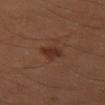follow-up: imaged on a skin check; not biopsied
subject: male, aged approximately 55
acquisition: 15 mm crop, total-body photography
lesion diameter: ≈3 mm
automated lesion analysis: a footprint of about 5 mm², a shape eccentricity near 0.5, and a shape-asymmetry score of about 0.25 (0 = symmetric); an automated nevus-likeness rating near 75 out of 100 and a lesion-detection confidence of about 100/100
anatomic site: the right thigh
lighting: cross-polarized illumination